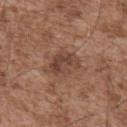Clinical impression:
The lesion was photographed on a routine skin check and not biopsied; there is no pathology result.
Image and clinical context:
This is a white-light tile. Located on the upper back. This image is a 15 mm lesion crop taken from a total-body photograph. Longest diameter approximately 4 mm. The patient is a male in their mid-50s.